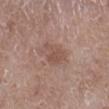Q: Was a biopsy performed?
A: total-body-photography surveillance lesion; no biopsy
Q: What is the anatomic site?
A: the right lower leg
Q: What kind of image is this?
A: 15 mm crop, total-body photography
Q: What are the patient's age and sex?
A: male, about 50 years old